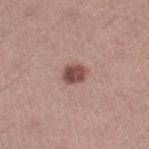Assessment:
Part of a total-body skin-imaging series; this lesion was reviewed on a skin check and was not flagged for biopsy.
Acquisition and patient details:
A 15 mm close-up extracted from a 3D total-body photography capture. A male subject aged approximately 40. On the right lower leg.